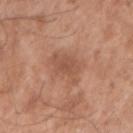Part of a total-body skin-imaging series; this lesion was reviewed on a skin check and was not flagged for biopsy. From the right upper arm. This is a white-light tile. A lesion tile, about 15 mm wide, cut from a 3D total-body photograph. Measured at roughly 5 mm in maximum diameter. A male subject aged around 50. The total-body-photography lesion software estimated a lesion area of about 8 mm², an eccentricity of roughly 0.9, and a shape-asymmetry score of about 0.35 (0 = symmetric). The analysis additionally found a mean CIELAB color near L≈52 a*≈22 b*≈31 and about 8 CIELAB-L* units darker than the surrounding skin. The analysis additionally found a border-irregularity index near 5/10 and peripheral color asymmetry of about 0.5. The software also gave a nevus-likeness score of about 0/100 and lesion-presence confidence of about 100/100.